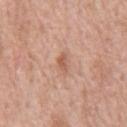Clinical impression:
The lesion was photographed on a routine skin check and not biopsied; there is no pathology result.
Context:
A 15 mm close-up tile from a total-body photography series done for melanoma screening. About 3 mm across. The lesion is on the chest. The subject is a male in their 60s.